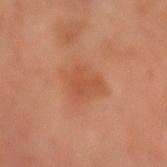{"image": {"source": "total-body photography crop", "field_of_view_mm": 15}, "automated_metrics": {"cielab_L": 53, "cielab_a": 27, "cielab_b": 36, "border_irregularity_0_10": 3.0, "color_variation_0_10": 2.0, "peripheral_color_asymmetry": 0.5, "lesion_detection_confidence_0_100": 100}, "lighting": "cross-polarized", "lesion_size": {"long_diameter_mm_approx": 4.0}, "site": "left arm", "patient": {"sex": "male", "age_approx": 50}}